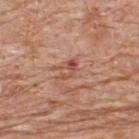The lesion's longest dimension is about 3 mm.
Located on the upper back.
Imaged with white-light lighting.
An algorithmic analysis of the crop reported a border-irregularity rating of about 5/10, a within-lesion color-variation index near 2.5/10, and radial color variation of about 1. The analysis additionally found an automated nevus-likeness rating near 0 out of 100 and a lesion-detection confidence of about 100/100.
A close-up tile cropped from a whole-body skin photograph, about 15 mm across.
A male patient, aged around 80.